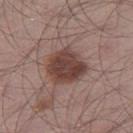Notes:
• notes: imaged on a skin check; not biopsied
• automated lesion analysis: a shape eccentricity near 0.55 and a symmetry-axis asymmetry near 0.2; a lesion color around L≈42 a*≈19 b*≈22 in CIELAB and a normalized border contrast of about 10; a border-irregularity index near 2/10 and a within-lesion color-variation index near 4/10
• size: ≈5 mm
• body site: the right thigh
• image source: 15 mm crop, total-body photography
• tile lighting: white-light illumination
• patient: male, roughly 55 years of age